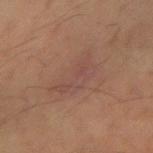Image and clinical context: A close-up tile cropped from a whole-body skin photograph, about 15 mm across. The patient is a male aged 53–57. The tile uses cross-polarized illumination. The lesion is located on the left forearm. About 5.5 mm across.